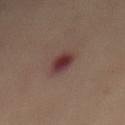No biopsy was performed on this lesion — it was imaged during a full skin examination and was not determined to be concerning. A roughly 15 mm field-of-view crop from a total-body skin photograph. A female patient, about 45 years old. About 4 mm across. This is a cross-polarized tile. From the mid back.